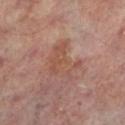| field | value |
|---|---|
| lesion diameter | ≈5 mm |
| location | the left lower leg |
| acquisition | total-body-photography crop, ~15 mm field of view |
| subject | male, aged approximately 65 |
| automated lesion analysis | a normalized border contrast of about 5.5; a border-irregularity index near 8/10, a within-lesion color-variation index near 3/10, and peripheral color asymmetry of about 1; an automated nevus-likeness rating near 0 out of 100 and a lesion-detection confidence of about 100/100 |